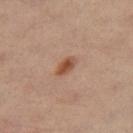{
  "biopsy_status": "not biopsied; imaged during a skin examination",
  "patient": {
    "sex": "female",
    "age_approx": 40
  },
  "lesion_size": {
    "long_diameter_mm_approx": 3.0
  },
  "site": "left leg",
  "image": {
    "source": "total-body photography crop",
    "field_of_view_mm": 15
  },
  "lighting": "cross-polarized",
  "automated_metrics": {
    "area_mm2_approx": 3.5,
    "eccentricity": 0.85,
    "shape_asymmetry": 0.2,
    "cielab_L": 50,
    "cielab_a": 22,
    "cielab_b": 32,
    "vs_skin_darker_L": 11.0,
    "vs_skin_contrast_norm": 9.0,
    "border_irregularity_0_10": 2.0,
    "color_variation_0_10": 4.0,
    "peripheral_color_asymmetry": 1.0
  }
}